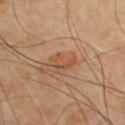| key | value |
|---|---|
| workup | catalogued during a skin exam; not biopsied |
| automated metrics | a footprint of about 4.5 mm², an outline eccentricity of about 0.8 (0 = round, 1 = elongated), and a symmetry-axis asymmetry near 0.5; border irregularity of about 5.5 on a 0–10 scale, a within-lesion color-variation index near 4/10, and peripheral color asymmetry of about 1.5; a nevus-likeness score of about 5/100 |
| lesion diameter | ≈3 mm |
| location | the chest |
| patient | male, in their 60s |
| illumination | cross-polarized |
| image | ~15 mm crop, total-body skin-cancer survey |